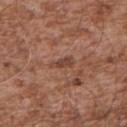{"biopsy_status": "not biopsied; imaged during a skin examination", "image": {"source": "total-body photography crop", "field_of_view_mm": 15}, "site": "upper back", "lighting": "white-light", "patient": {"sex": "male", "age_approx": 55}}